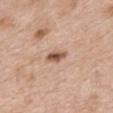Imaged during a routine full-body skin examination; the lesion was not biopsied and no histopathology is available. On the mid back. The subject is a female aged around 40. This is a white-light tile. A region of skin cropped from a whole-body photographic capture, roughly 15 mm wide.